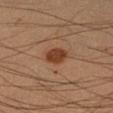The lesion was tiled from a total-body skin photograph and was not biopsied.
A close-up tile cropped from a whole-body skin photograph, about 15 mm across.
A male patient, in their mid-30s.
The lesion's longest dimension is about 3 mm.
This is a cross-polarized tile.
From the right forearm.
The total-body-photography lesion software estimated a shape eccentricity near 0.65 and two-axis asymmetry of about 0.2. It also reported a lesion color around L≈35 a*≈21 b*≈29 in CIELAB, about 10 CIELAB-L* units darker than the surrounding skin, and a lesion-to-skin contrast of about 9.5 (normalized; higher = more distinct). The analysis additionally found border irregularity of about 1.5 on a 0–10 scale, a within-lesion color-variation index near 1.5/10, and radial color variation of about 0.5. The analysis additionally found an automated nevus-likeness rating near 95 out of 100.